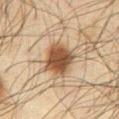Impression: This lesion was catalogued during total-body skin photography and was not selected for biopsy. Background: From the abdomen. This is a cross-polarized tile. A roughly 15 mm field-of-view crop from a total-body skin photograph. A male patient aged 63–67. Longest diameter approximately 4 mm.